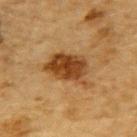This lesion was catalogued during total-body skin photography and was not selected for biopsy.
The subject is a male in their mid- to late 80s or older.
A roughly 15 mm field-of-view crop from a total-body skin photograph.
On the back.